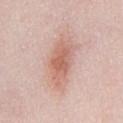Notes:
– biopsy status: imaged on a skin check; not biopsied
– image: total-body-photography crop, ~15 mm field of view
– size: ≈6 mm
– site: the chest
– tile lighting: white-light
– subject: male, about 25 years old